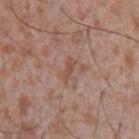Assessment:
The lesion was photographed on a routine skin check and not biopsied; there is no pathology result.
Acquisition and patient details:
This image is a 15 mm lesion crop taken from a total-body photograph. A male patient aged 43–47. Located on the chest. Imaged with white-light lighting.